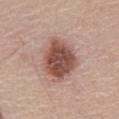Impression: The lesion was tiled from a total-body skin photograph and was not biopsied. Acquisition and patient details: A close-up tile cropped from a whole-body skin photograph, about 15 mm across. From the front of the torso. Automated tile analysis of the lesion measured a lesion area of about 19 mm², an outline eccentricity of about 0.6 (0 = round, 1 = elongated), and a shape-asymmetry score of about 0.2 (0 = symmetric). About 5.5 mm across. This is a white-light tile. A male subject, aged 58 to 62.